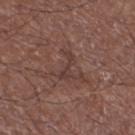Q: Was a biopsy performed?
A: total-body-photography surveillance lesion; no biopsy
Q: What lighting was used for the tile?
A: white-light
Q: Who is the patient?
A: male, about 65 years old
Q: What kind of image is this?
A: ~15 mm crop, total-body skin-cancer survey
Q: What is the lesion's diameter?
A: about 3.5 mm
Q: What is the anatomic site?
A: the left lower leg